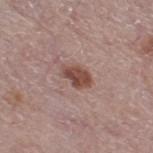Q: Was a biopsy performed?
A: imaged on a skin check; not biopsied
Q: What lighting was used for the tile?
A: white-light illumination
Q: Patient demographics?
A: female, about 65 years old
Q: What kind of image is this?
A: 15 mm crop, total-body photography
Q: Where on the body is the lesion?
A: the right thigh